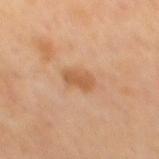image = 15 mm crop, total-body photography | lighting = cross-polarized | anatomic site = the mid back | patient = male, aged around 70 | automated lesion analysis = a lesion color around L≈55 a*≈21 b*≈37 in CIELAB, roughly 9 lightness units darker than nearby skin, and a normalized lesion–skin contrast near 7; a border-irregularity rating of about 2.5/10 and radial color variation of about 0.5; an automated nevus-likeness rating near 35 out of 100 and a detector confidence of about 100 out of 100 that the crop contains a lesion.